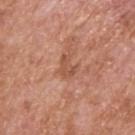No biopsy was performed on this lesion — it was imaged during a full skin examination and was not determined to be concerning. The patient is a male approximately 65 years of age. About 3 mm across. Cropped from a whole-body photographic skin survey; the tile spans about 15 mm. The tile uses white-light illumination. On the back.